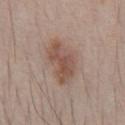The lesion is located on the chest. A male subject aged approximately 40. Longest diameter approximately 5 mm. Cropped from a whole-body photographic skin survey; the tile spans about 15 mm.